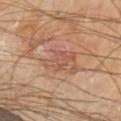Recorded during total-body skin imaging; not selected for excision or biopsy.
From the left upper arm.
Captured under cross-polarized illumination.
The patient is a male aged 63 to 67.
Automated tile analysis of the lesion measured a lesion area of about 5.5 mm², a shape eccentricity near 0.75, and a symmetry-axis asymmetry near 0.45. And it measured about 7 CIELAB-L* units darker than the surrounding skin. The analysis additionally found a border-irregularity rating of about 6/10, internal color variation of about 2 on a 0–10 scale, and a peripheral color-asymmetry measure near 0.5.
A region of skin cropped from a whole-body photographic capture, roughly 15 mm wide.
The lesion's longest dimension is about 3.5 mm.